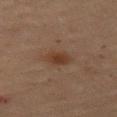Q: What is the lesion's diameter?
A: about 3 mm
Q: Patient demographics?
A: female, in their 70s
Q: What kind of image is this?
A: ~15 mm crop, total-body skin-cancer survey
Q: What is the anatomic site?
A: the leg
Q: How was the tile lit?
A: cross-polarized